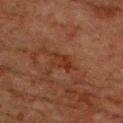{
  "biopsy_status": "not biopsied; imaged during a skin examination",
  "site": "right upper arm",
  "image": {
    "source": "total-body photography crop",
    "field_of_view_mm": 15
  },
  "patient": {
    "sex": "male",
    "age_approx": 80
  },
  "lesion_size": {
    "long_diameter_mm_approx": 4.0
  },
  "lighting": "cross-polarized"
}